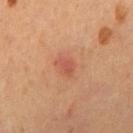  biopsy_status: not biopsied; imaged during a skin examination
  automated_metrics:
    cielab_L: 49
    cielab_a: 26
    cielab_b: 30
    vs_skin_contrast_norm: 5.5
    nevus_likeness_0_100: 5
  site: chest
  image:
    source: total-body photography crop
    field_of_view_mm: 15
  lesion_size:
    long_diameter_mm_approx: 2.5
  patient:
    sex: male
    age_approx: 55
  lighting: cross-polarized